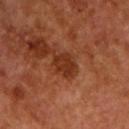Q: Is there a histopathology result?
A: imaged on a skin check; not biopsied
Q: What lighting was used for the tile?
A: cross-polarized illumination
Q: What is the lesion's diameter?
A: ~3 mm (longest diameter)
Q: Patient demographics?
A: male, aged approximately 55
Q: What is the imaging modality?
A: 15 mm crop, total-body photography
Q: What is the anatomic site?
A: the chest